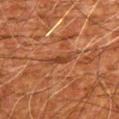Notes:
* notes: no biopsy performed (imaged during a skin exam)
* site: the left upper arm
* image source: ~15 mm crop, total-body skin-cancer survey
* patient: male, about 80 years old